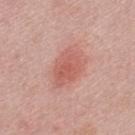<record>
<biopsy_status>not biopsied; imaged during a skin examination</biopsy_status>
<patient>
  <sex>male</sex>
  <age_approx>45</age_approx>
</patient>
<image>
  <source>total-body photography crop</source>
  <field_of_view_mm>15</field_of_view_mm>
</image>
<site>back</site>
</record>